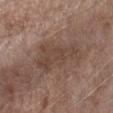| key | value |
|---|---|
| workup | catalogued during a skin exam; not biopsied |
| subject | male, in their 70s |
| automated metrics | an area of roughly 17 mm², an eccentricity of roughly 0.9, and a symmetry-axis asymmetry near 0.4; a nevus-likeness score of about 0/100 |
| illumination | white-light |
| location | the left forearm |
| image | ~15 mm crop, total-body skin-cancer survey |
| diameter | about 7 mm |